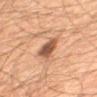workup — catalogued during a skin exam; not biopsied
patient — male, aged 58–62
site — the mid back
illumination — cross-polarized
image — ~15 mm tile from a whole-body skin photo
TBP lesion metrics — a lesion area of about 5.5 mm², an eccentricity of roughly 0.8, and a shape-asymmetry score of about 0.3 (0 = symmetric); an average lesion color of about L≈39 a*≈18 b*≈25 (CIELAB)